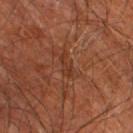The lesion was photographed on a routine skin check and not biopsied; there is no pathology result.
The lesion is located on the right leg.
Imaged with cross-polarized lighting.
Automated image analysis of the tile measured an area of roughly 3 mm², a shape eccentricity near 0.9, and a shape-asymmetry score of about 0.35 (0 = symmetric).
Cropped from a total-body skin-imaging series; the visible field is about 15 mm.
Measured at roughly 3 mm in maximum diameter.
A male subject about 60 years old.